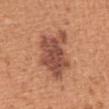This lesion was catalogued during total-body skin photography and was not selected for biopsy.
A male subject, approximately 55 years of age.
From the abdomen.
The lesion's longest dimension is about 7 mm.
A 15 mm crop from a total-body photograph taken for skin-cancer surveillance.
Captured under white-light illumination.